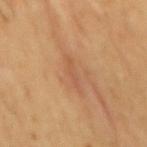This lesion was catalogued during total-body skin photography and was not selected for biopsy.
Cropped from a total-body skin-imaging series; the visible field is about 15 mm.
Captured under cross-polarized illumination.
Located on the mid back.
The patient is a male aged 58–62.